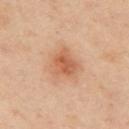A female patient aged around 40.
Approximately 3.5 mm at its widest.
Captured under cross-polarized illumination.
The lesion is located on the arm.
A lesion tile, about 15 mm wide, cut from a 3D total-body photograph.